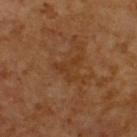Measured at roughly 3 mm in maximum diameter. The total-body-photography lesion software estimated a footprint of about 4 mm², a shape eccentricity near 0.6, and two-axis asymmetry of about 0.8. The software also gave a mean CIELAB color near L≈33 a*≈20 b*≈31, about 5 CIELAB-L* units darker than the surrounding skin, and a lesion-to-skin contrast of about 5 (normalized; higher = more distinct). And it measured a border-irregularity index near 9/10, a within-lesion color-variation index near 0/10, and radial color variation of about 0. And it measured a classifier nevus-likeness of about 0/100 and a lesion-detection confidence of about 100/100. A male subject about 60 years old. A region of skin cropped from a whole-body photographic capture, roughly 15 mm wide. Imaged with cross-polarized lighting. From the upper back.